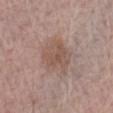This lesion was catalogued during total-body skin photography and was not selected for biopsy.
Located on the right forearm.
A roughly 15 mm field-of-view crop from a total-body skin photograph.
The subject is a female about 70 years old.
Captured under white-light illumination.